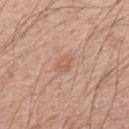Clinical summary: A 15 mm close-up tile from a total-body photography series done for melanoma screening. Captured under white-light illumination. A male subject aged approximately 55. The lesion is located on the upper back.